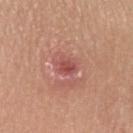Assessment:
Captured during whole-body skin photography for melanoma surveillance; the lesion was not biopsied.
Clinical summary:
A female patient, aged 43 to 47. From the left upper arm. A close-up tile cropped from a whole-body skin photograph, about 15 mm across. The total-body-photography lesion software estimated a footprint of about 4 mm², an outline eccentricity of about 0.6 (0 = round, 1 = elongated), and a shape-asymmetry score of about 0.25 (0 = symmetric). And it measured a mean CIELAB color near L≈51 a*≈29 b*≈26, about 9 CIELAB-L* units darker than the surrounding skin, and a lesion-to-skin contrast of about 6.5 (normalized; higher = more distinct). The software also gave a border-irregularity index near 2.5/10, a color-variation rating of about 4/10, and radial color variation of about 1.5. The analysis additionally found a classifier nevus-likeness of about 0/100 and a detector confidence of about 100 out of 100 that the crop contains a lesion.